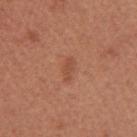Assessment:
The lesion was tiled from a total-body skin photograph and was not biopsied.